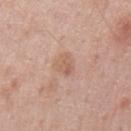• follow-up · total-body-photography surveillance lesion; no biopsy
• body site · the mid back
• patient · male, in their mid- to late 50s
• diameter · ~3 mm (longest diameter)
• tile lighting · white-light illumination
• image · ~15 mm crop, total-body skin-cancer survey